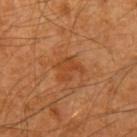image-analysis metrics: a footprint of about 6 mm², a shape eccentricity near 0.55, and a shape-asymmetry score of about 0.5 (0 = symmetric); an average lesion color of about L≈44 a*≈27 b*≈39 (CIELAB), about 7 CIELAB-L* units darker than the surrounding skin, and a normalized lesion–skin contrast near 6 | image source: 15 mm crop, total-body photography | location: the left upper arm | lighting: cross-polarized | lesion size: about 3 mm | subject: male, about 60 years old.